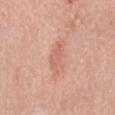workup: imaged on a skin check; not biopsied | size: ~4.5 mm (longest diameter) | location: the mid back | patient: male, aged 78–82 | image: total-body-photography crop, ~15 mm field of view | tile lighting: white-light.